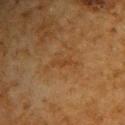Clinical impression: Recorded during total-body skin imaging; not selected for excision or biopsy. Image and clinical context: The lesion's longest dimension is about 2.5 mm. The lesion-visualizer software estimated a lesion area of about 2 mm², an eccentricity of roughly 0.95, and a symmetry-axis asymmetry near 0.4. The analysis additionally found a lesion color around L≈34 a*≈18 b*≈32 in CIELAB, about 5 CIELAB-L* units darker than the surrounding skin, and a lesion-to-skin contrast of about 5 (normalized; higher = more distinct). The analysis additionally found a border-irregularity index near 4.5/10 and a within-lesion color-variation index near 0/10. And it measured a classifier nevus-likeness of about 0/100 and a lesion-detection confidence of about 100/100. A male subject, in their 60s. A 15 mm close-up tile from a total-body photography series done for melanoma screening. This is a cross-polarized tile. The lesion is on the upper back.